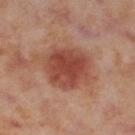Part of a total-body skin-imaging series; this lesion was reviewed on a skin check and was not flagged for biopsy. A female subject about 55 years old. This is a cross-polarized tile. The lesion is located on the leg. Approximately 5.5 mm at its widest. A lesion tile, about 15 mm wide, cut from a 3D total-body photograph.